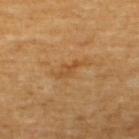biopsy status: catalogued during a skin exam; not biopsied
acquisition: ~15 mm tile from a whole-body skin photo
automated lesion analysis: roughly 6 lightness units darker than nearby skin and a normalized border contrast of about 5.5
location: the upper back
tile lighting: cross-polarized illumination
subject: male, roughly 60 years of age
diameter: ~4 mm (longest diameter)